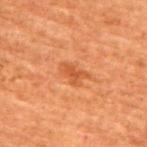No biopsy was performed on this lesion — it was imaged during a full skin examination and was not determined to be concerning. On the front of the torso. A female patient, aged 78–82. A 15 mm crop from a total-body photograph taken for skin-cancer surveillance.